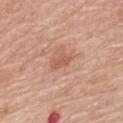{"biopsy_status": "not biopsied; imaged during a skin examination", "patient": {"sex": "female", "age_approx": 65}, "site": "upper back", "image": {"source": "total-body photography crop", "field_of_view_mm": 15}}